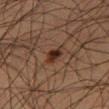Assessment:
This lesion was catalogued during total-body skin photography and was not selected for biopsy.
Image and clinical context:
A male patient aged approximately 50. On the right thigh. A close-up tile cropped from a whole-body skin photograph, about 15 mm across.